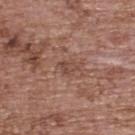Recorded during total-body skin imaging; not selected for excision or biopsy.
On the upper back.
The tile uses white-light illumination.
A female subject, approximately 65 years of age.
A 15 mm close-up tile from a total-body photography series done for melanoma screening.
About 3 mm across.
An algorithmic analysis of the crop reported a footprint of about 3 mm² and a shape eccentricity near 0.8. The software also gave a lesion-to-skin contrast of about 6 (normalized; higher = more distinct).